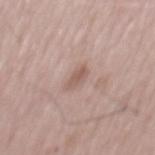follow-up: no biopsy performed (imaged during a skin exam) | image source: ~15 mm crop, total-body skin-cancer survey | automated metrics: a lesion area of about 3 mm² and a shape-asymmetry score of about 0.3 (0 = symmetric); a border-irregularity rating of about 3/10 and peripheral color asymmetry of about 0; a nevus-likeness score of about 0/100 and a lesion-detection confidence of about 100/100 | lesion diameter: about 3 mm | subject: male, roughly 55 years of age.